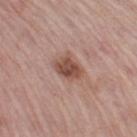biopsy_status: not biopsied; imaged during a skin examination
image:
  source: total-body photography crop
  field_of_view_mm: 15
lighting: white-light
patient:
  sex: male
  age_approx: 60
automated_metrics:
  eccentricity: 0.6
  cielab_L: 50
  cielab_a: 21
  cielab_b: 26
  vs_skin_darker_L: 12.0
  vs_skin_contrast_norm: 9.0
  border_irregularity_0_10: 2.0
  color_variation_0_10: 3.5
lesion_size:
  long_diameter_mm_approx: 3.0
site: right thigh